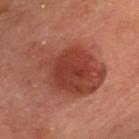This lesion was catalogued during total-body skin photography and was not selected for biopsy.
The lesion is on the head or neck.
A lesion tile, about 15 mm wide, cut from a 3D total-body photograph.
The recorded lesion diameter is about 9 mm.
A female patient approximately 60 years of age.
Imaged with cross-polarized lighting.
Automated tile analysis of the lesion measured a lesion area of about 35 mm², an outline eccentricity of about 0.75 (0 = round, 1 = elongated), and a shape-asymmetry score of about 0.3 (0 = symmetric). And it measured a border-irregularity rating of about 4/10 and a color-variation rating of about 4.5/10. It also reported an automated nevus-likeness rating near 80 out of 100 and a detector confidence of about 100 out of 100 that the crop contains a lesion.